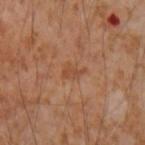{
  "lesion_size": {
    "long_diameter_mm_approx": 3.0
  },
  "image": {
    "source": "total-body photography crop",
    "field_of_view_mm": 15
  },
  "automated_metrics": {
    "cielab_L": 48,
    "cielab_a": 23,
    "cielab_b": 33,
    "vs_skin_darker_L": 6.0,
    "nevus_likeness_0_100": 0,
    "lesion_detection_confidence_0_100": 100
  },
  "site": "right forearm",
  "patient": {
    "sex": "male",
    "age_approx": 55
  },
  "lighting": "cross-polarized"
}